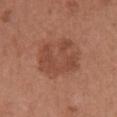biopsy_status: not biopsied; imaged during a skin examination
image:
  source: total-body photography crop
  field_of_view_mm: 15
patient:
  sex: female
  age_approx: 65
lighting: white-light
lesion_size:
  long_diameter_mm_approx: 5.5
site: chest